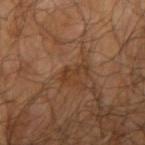Q: Is there a histopathology result?
A: catalogued during a skin exam; not biopsied
Q: Who is the patient?
A: male, approximately 65 years of age
Q: What kind of image is this?
A: total-body-photography crop, ~15 mm field of view
Q: Illumination type?
A: cross-polarized illumination
Q: Lesion location?
A: the right upper arm
Q: Automated lesion metrics?
A: a lesion area of about 3 mm²; a mean CIELAB color near L≈34 a*≈19 b*≈30, roughly 6 lightness units darker than nearby skin, and a lesion-to-skin contrast of about 6.5 (normalized; higher = more distinct); a border-irregularity rating of about 7/10, a color-variation rating of about 0/10, and peripheral color asymmetry of about 0; a nevus-likeness score of about 0/100